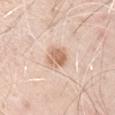workup: total-body-photography surveillance lesion; no biopsy | location: the arm | image source: ~15 mm tile from a whole-body skin photo | patient: male, aged 68–72 | lesion size: about 2.5 mm | automated metrics: an area of roughly 6 mm² and two-axis asymmetry of about 0.25; a lesion color around L≈66 a*≈19 b*≈32 in CIELAB and a normalized border contrast of about 8; a classifier nevus-likeness of about 60/100 | tile lighting: white-light illumination.